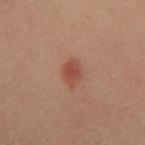• location: the chest
• automated metrics: a border-irregularity rating of about 2/10, a within-lesion color-variation index near 1.5/10, and a peripheral color-asymmetry measure near 0.5; a classifier nevus-likeness of about 100/100 and a lesion-detection confidence of about 100/100
• lighting: cross-polarized
• subject: male, aged 38 to 42
• acquisition: 15 mm crop, total-body photography